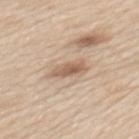Captured during whole-body skin photography for melanoma surveillance; the lesion was not biopsied. A 15 mm crop from a total-body photograph taken for skin-cancer surveillance. The lesion is on the mid back. The patient is a male aged 58–62. About 3.5 mm across. An algorithmic analysis of the crop reported an area of roughly 5.5 mm², an outline eccentricity of about 0.9 (0 = round, 1 = elongated), and a symmetry-axis asymmetry near 0.3.